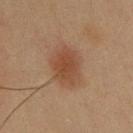follow-up: imaged on a skin check; not biopsied | imaging modality: ~15 mm tile from a whole-body skin photo | image-analysis metrics: a lesion area of about 13 mm² and a shape-asymmetry score of about 0.15 (0 = symmetric); border irregularity of about 1.5 on a 0–10 scale, a color-variation rating of about 3/10, and a peripheral color-asymmetry measure near 1; a nevus-likeness score of about 95/100 and a detector confidence of about 100 out of 100 that the crop contains a lesion | illumination: cross-polarized | location: the upper back | size: about 4.5 mm | patient: male, in their mid-30s.